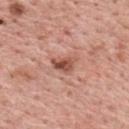Q: Was a biopsy performed?
A: no biopsy performed (imaged during a skin exam)
Q: Lesion location?
A: the upper back
Q: Who is the patient?
A: male, aged 58–62
Q: What lighting was used for the tile?
A: white-light
Q: What is the imaging modality?
A: ~15 mm crop, total-body skin-cancer survey